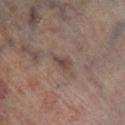The lesion was photographed on a routine skin check and not biopsied; there is no pathology result. The lesion is located on the leg. About 2.5 mm across. A male patient roughly 65 years of age. A 15 mm close-up tile from a total-body photography series done for melanoma screening. Captured under cross-polarized illumination.